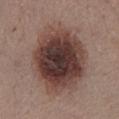<lesion>
  <biopsy_status>not biopsied; imaged during a skin examination</biopsy_status>
  <lesion_size>
    <long_diameter_mm_approx>9.5</long_diameter_mm_approx>
  </lesion_size>
  <image>
    <source>total-body photography crop</source>
    <field_of_view_mm>15</field_of_view_mm>
  </image>
  <lighting>white-light</lighting>
  <site>chest</site>
  <patient>
    <sex>female</sex>
    <age_approx>50</age_approx>
  </patient>
</lesion>